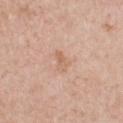lesion diameter=about 2.5 mm; tile lighting=white-light illumination; image source=~15 mm tile from a whole-body skin photo; site=the chest; patient=female, aged 38–42.